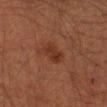* workup · total-body-photography surveillance lesion; no biopsy
* automated lesion analysis · border irregularity of about 2.5 on a 0–10 scale; a detector confidence of about 100 out of 100 that the crop contains a lesion
* subject · male, in their mid- to late 40s
* size · ~3 mm (longest diameter)
* acquisition · ~15 mm tile from a whole-body skin photo
* anatomic site · the right upper arm